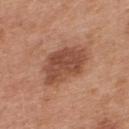{
  "biopsy_status": "not biopsied; imaged during a skin examination",
  "site": "back",
  "lighting": "white-light",
  "patient": {
    "sex": "male",
    "age_approx": 30
  },
  "image": {
    "source": "total-body photography crop",
    "field_of_view_mm": 15
  }
}